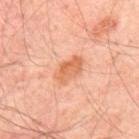<tbp_lesion>
  <biopsy_status>not biopsied; imaged during a skin examination</biopsy_status>
  <site>upper back</site>
  <lighting>cross-polarized</lighting>
  <image>
    <source>total-body photography crop</source>
    <field_of_view_mm>15</field_of_view_mm>
  </image>
  <automated_metrics>
    <vs_skin_darker_L>10.0</vs_skin_darker_L>
    <vs_skin_contrast_norm>7.5</vs_skin_contrast_norm>
    <color_variation_0_10>3.5</color_variation_0_10>
    <nevus_likeness_0_100>80</nevus_likeness_0_100>
    <lesion_detection_confidence_0_100>100</lesion_detection_confidence_0_100>
  </automated_metrics>
  <patient>
    <age_approx>55</age_approx>
  </patient>
  <lesion_size>
    <long_diameter_mm_approx>3.5</long_diameter_mm_approx>
  </lesion_size>
</tbp_lesion>